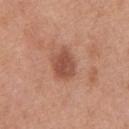Q: Was this lesion biopsied?
A: no biopsy performed (imaged during a skin exam)
Q: What is the anatomic site?
A: the front of the torso
Q: What are the patient's age and sex?
A: male, aged 68 to 72
Q: How was the tile lit?
A: white-light
Q: What is the imaging modality?
A: total-body-photography crop, ~15 mm field of view
Q: Lesion size?
A: ≈3.5 mm